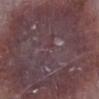No biopsy was performed on this lesion — it was imaged during a full skin examination and was not determined to be concerning.
A male patient, in their mid-70s.
Cropped from a whole-body photographic skin survey; the tile spans about 15 mm.
The lesion is on the left lower leg.